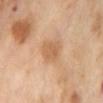<tbp_lesion>
  <biopsy_status>not biopsied; imaged during a skin examination</biopsy_status>
  <site>abdomen</site>
  <patient>
    <sex>female</sex>
    <age_approx>55</age_approx>
  </patient>
  <automated_metrics>
    <eccentricity>0.7</eccentricity>
    <shape_asymmetry>0.2</shape_asymmetry>
    <cielab_L>61</cielab_L>
    <cielab_a>21</cielab_a>
    <cielab_b>36</cielab_b>
    <vs_skin_darker_L>9.0</vs_skin_darker_L>
    <vs_skin_contrast_norm>6.0</vs_skin_contrast_norm>
  </automated_metrics>
  <image>
    <source>total-body photography crop</source>
    <field_of_view_mm>15</field_of_view_mm>
  </image>
  <lesion_size>
    <long_diameter_mm_approx>3.0</long_diameter_mm_approx>
  </lesion_size>
  <lighting>cross-polarized</lighting>
</tbp_lesion>